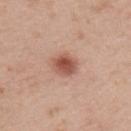Q: Is there a histopathology result?
A: no biopsy performed (imaged during a skin exam)
Q: How was this image acquired?
A: total-body-photography crop, ~15 mm field of view
Q: How large is the lesion?
A: ~3.5 mm (longest diameter)
Q: What lighting was used for the tile?
A: white-light
Q: Lesion location?
A: the upper back
Q: Patient demographics?
A: male, aged around 40